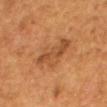From the mid back. The patient is a female about 50 years old. Measured at roughly 6 mm in maximum diameter. A region of skin cropped from a whole-body photographic capture, roughly 15 mm wide.